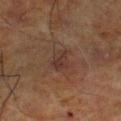Findings:
• image source · ~15 mm crop, total-body skin-cancer survey
• subject · male, approximately 70 years of age
• lesion diameter · ≈3 mm
• anatomic site · the leg
• illumination · cross-polarized
• automated metrics · roughly 5 lightness units darker than nearby skin; a border-irregularity rating of about 3/10, a within-lesion color-variation index near 2.5/10, and peripheral color asymmetry of about 1; a classifier nevus-likeness of about 5/100 and a detector confidence of about 100 out of 100 that the crop contains a lesion